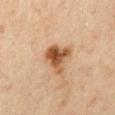Imaged during a routine full-body skin examination; the lesion was not biopsied and no histopathology is available. Located on the mid back. A 15 mm crop from a total-body photograph taken for skin-cancer surveillance. A male patient about 45 years old. This is a cross-polarized tile.